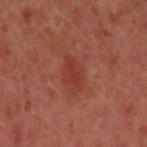Assessment: Part of a total-body skin-imaging series; this lesion was reviewed on a skin check and was not flagged for biopsy. Context: A close-up tile cropped from a whole-body skin photograph, about 15 mm across. About 2.5 mm across. A female patient in their 40s. The tile uses cross-polarized illumination. From the left upper arm.